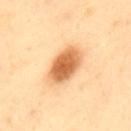This lesion was catalogued during total-body skin photography and was not selected for biopsy. The lesion is on the upper back. A region of skin cropped from a whole-body photographic capture, roughly 15 mm wide. A male subject aged approximately 40.